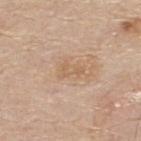<lesion>
<biopsy_status>not biopsied; imaged during a skin examination</biopsy_status>
<site>upper back</site>
<patient>
  <sex>male</sex>
  <age_approx>80</age_approx>
</patient>
<image>
  <source>total-body photography crop</source>
  <field_of_view_mm>15</field_of_view_mm>
</image>
</lesion>